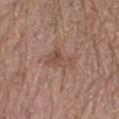{"biopsy_status": "not biopsied; imaged during a skin examination", "image": {"source": "total-body photography crop", "field_of_view_mm": 15}, "lesion_size": {"long_diameter_mm_approx": 4.5}, "automated_metrics": {"cielab_L": 49, "cielab_a": 19, "cielab_b": 26, "vs_skin_darker_L": 7.0, "vs_skin_contrast_norm": 5.5}, "patient": {"sex": "male", "age_approx": 20}, "site": "right lower leg", "lighting": "white-light"}